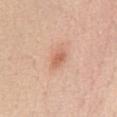Notes:
* biopsy status · imaged on a skin check; not biopsied
* anatomic site · the abdomen
* automated metrics · a lesion area of about 4 mm², a shape eccentricity near 0.8, and a shape-asymmetry score of about 0.3 (0 = symmetric); a lesion color around L≈63 a*≈24 b*≈32 in CIELAB, about 10 CIELAB-L* units darker than the surrounding skin, and a lesion-to-skin contrast of about 6.5 (normalized; higher = more distinct); a border-irregularity index near 2.5/10, a within-lesion color-variation index near 2/10, and a peripheral color-asymmetry measure near 0.5
* subject · female, in their 40s
* image source · ~15 mm tile from a whole-body skin photo
* lighting · white-light illumination